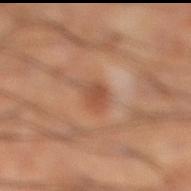notes: no biopsy performed (imaged during a skin exam)
site: the right lower leg
patient: male, aged 58–62
image: ~15 mm crop, total-body skin-cancer survey
tile lighting: cross-polarized illumination